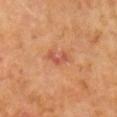Imaged during a routine full-body skin examination; the lesion was not biopsied and no histopathology is available.
The patient is a male aged 58 to 62.
The lesion is on the right arm.
Cropped from a total-body skin-imaging series; the visible field is about 15 mm.